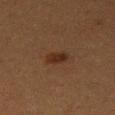| feature | finding |
|---|---|
| biopsy status | no biopsy performed (imaged during a skin exam) |
| diameter | ≈2.5 mm |
| site | the right thigh |
| subject | female, in their 40s |
| image source | total-body-photography crop, ~15 mm field of view |
| illumination | cross-polarized |